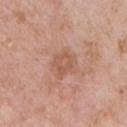Assessment: Recorded during total-body skin imaging; not selected for excision or biopsy. Clinical summary: The tile uses white-light illumination. An algorithmic analysis of the crop reported a footprint of about 5 mm², a shape eccentricity near 0.4, and a shape-asymmetry score of about 0.2 (0 = symmetric). The software also gave a border-irregularity index near 2/10, a within-lesion color-variation index near 3/10, and peripheral color asymmetry of about 1. The patient is a male aged 58 to 62. A region of skin cropped from a whole-body photographic capture, roughly 15 mm wide. From the chest.